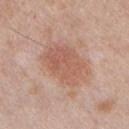Impression: Part of a total-body skin-imaging series; this lesion was reviewed on a skin check and was not flagged for biopsy. Image and clinical context: Measured at roughly 6 mm in maximum diameter. A male subject, aged 33 to 37. A 15 mm close-up tile from a total-body photography series done for melanoma screening. The lesion is on the chest.